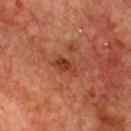workup = total-body-photography surveillance lesion; no biopsy